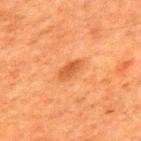The lesion was photographed on a routine skin check and not biopsied; there is no pathology result. The lesion is located on the upper back. This image is a 15 mm lesion crop taken from a total-body photograph. A male subject about 60 years old.